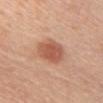Captured during whole-body skin photography for melanoma surveillance; the lesion was not biopsied.
Imaged with white-light lighting.
About 4 mm across.
A female patient aged 63–67.
Automated image analysis of the tile measured a lesion area of about 11 mm² and an eccentricity of roughly 0.45. It also reported a nevus-likeness score of about 100/100.
From the chest.
Cropped from a total-body skin-imaging series; the visible field is about 15 mm.